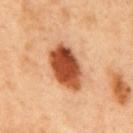About 6 mm across. Cropped from a whole-body photographic skin survey; the tile spans about 15 mm. The tile uses cross-polarized illumination. A male subject, aged 48–52. The lesion is located on the mid back.